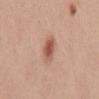Clinical impression: The lesion was tiled from a total-body skin photograph and was not biopsied. Image and clinical context: A female patient, roughly 50 years of age. This image is a 15 mm lesion crop taken from a total-body photograph. On the mid back.